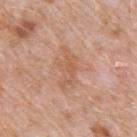Captured during whole-body skin photography for melanoma surveillance; the lesion was not biopsied.